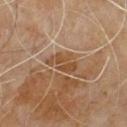Case summary:
* follow-up — imaged on a skin check; not biopsied
* TBP lesion metrics — about 8 CIELAB-L* units darker than the surrounding skin; a border-irregularity rating of about 4.5/10, internal color variation of about 3 on a 0–10 scale, and radial color variation of about 1
* illumination — cross-polarized illumination
* subject — male, aged approximately 60
* image — 15 mm crop, total-body photography
* diameter — about 4 mm
* body site — the upper back